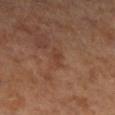follow-up=imaged on a skin check; not biopsied | body site=the right lower leg | subject=female, approximately 65 years of age | diameter=~2.5 mm (longest diameter) | acquisition=15 mm crop, total-body photography.